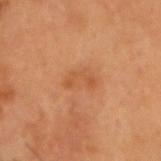Q: Is there a histopathology result?
A: no biopsy performed (imaged during a skin exam)
Q: What kind of image is this?
A: ~15 mm tile from a whole-body skin photo
Q: Who is the patient?
A: male, approximately 55 years of age
Q: Lesion location?
A: the head or neck
Q: How was the tile lit?
A: cross-polarized illumination